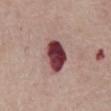• notes: catalogued during a skin exam; not biopsied
• subject: male, in their mid-80s or older
• illumination: white-light
• lesion size: ~4 mm (longest diameter)
• acquisition: 15 mm crop, total-body photography
• site: the abdomen
• image-analysis metrics: a lesion area of about 11 mm² and an outline eccentricity of about 0.65 (0 = round, 1 = elongated); a lesion color around L≈40 a*≈28 b*≈17 in CIELAB and a normalized lesion–skin contrast near 16.5